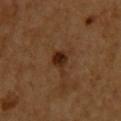notes: total-body-photography surveillance lesion; no biopsy
automated metrics: a footprint of about 5.5 mm², a shape eccentricity near 0.7, and a shape-asymmetry score of about 0.5 (0 = symmetric)
location: the upper back
tile lighting: cross-polarized illumination
image: ~15 mm tile from a whole-body skin photo
patient: female, approximately 55 years of age